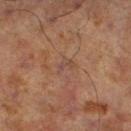Q: Was this lesion biopsied?
A: imaged on a skin check; not biopsied
Q: Where on the body is the lesion?
A: the left lower leg
Q: What kind of image is this?
A: total-body-photography crop, ~15 mm field of view
Q: Patient demographics?
A: male, roughly 70 years of age